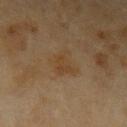The lesion is on the left upper arm. This image is a 15 mm lesion crop taken from a total-body photograph. Longest diameter approximately 3.5 mm. Automated tile analysis of the lesion measured an average lesion color of about L≈38 a*≈14 b*≈30 (CIELAB) and roughly 4 lightness units darker than nearby skin. It also reported a border-irregularity rating of about 4.5/10, internal color variation of about 2.5 on a 0–10 scale, and peripheral color asymmetry of about 1. A female subject in their 60s. Captured under cross-polarized illumination.